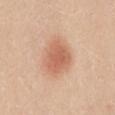Imaged during a routine full-body skin examination; the lesion was not biopsied and no histopathology is available.
Automated image analysis of the tile measured an area of roughly 12 mm² and two-axis asymmetry of about 0.2. The analysis additionally found a lesion color around L≈62 a*≈24 b*≈33 in CIELAB. The software also gave border irregularity of about 2 on a 0–10 scale and radial color variation of about 0.5. It also reported an automated nevus-likeness rating near 100 out of 100 and a lesion-detection confidence of about 100/100.
A female patient aged 18–22.
On the mid back.
The tile uses white-light illumination.
Longest diameter approximately 4.5 mm.
A 15 mm crop from a total-body photograph taken for skin-cancer surveillance.